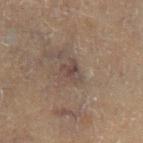Part of a total-body skin-imaging series; this lesion was reviewed on a skin check and was not flagged for biopsy. Cropped from a whole-body photographic skin survey; the tile spans about 15 mm. The lesion-visualizer software estimated a lesion area of about 3.5 mm², an eccentricity of roughly 0.6, and a symmetry-axis asymmetry near 0.4. The software also gave an average lesion color of about L≈32 a*≈11 b*≈16 (CIELAB) and a lesion-to-skin contrast of about 6.5 (normalized; higher = more distinct). A male patient, roughly 70 years of age. Captured under cross-polarized illumination. On the left lower leg.